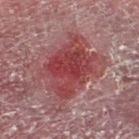Case summary:
* follow-up — imaged on a skin check; not biopsied
* subject — male, approximately 55 years of age
* anatomic site — the right thigh
* acquisition — ~15 mm crop, total-body skin-cancer survey